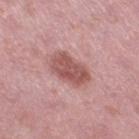{
  "biopsy_status": "not biopsied; imaged during a skin examination",
  "site": "right thigh",
  "lesion_size": {
    "long_diameter_mm_approx": 4.5
  },
  "image": {
    "source": "total-body photography crop",
    "field_of_view_mm": 15
  },
  "patient": {
    "sex": "female",
    "age_approx": 40
  },
  "automated_metrics": {
    "border_irregularity_0_10": 2.0,
    "color_variation_0_10": 3.5,
    "peripheral_color_asymmetry": 1.0,
    "lesion_detection_confidence_0_100": 100
  }
}